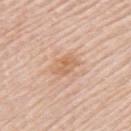Assessment: Imaged during a routine full-body skin examination; the lesion was not biopsied and no histopathology is available. Acquisition and patient details: A female subject aged 63–67. Measured at roughly 3.5 mm in maximum diameter. The lesion is located on the left upper arm. The total-body-photography lesion software estimated a lesion area of about 6 mm² and a shape eccentricity near 0.75. Captured under white-light illumination. This image is a 15 mm lesion crop taken from a total-body photograph.